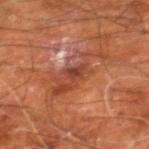Assessment: Part of a total-body skin-imaging series; this lesion was reviewed on a skin check and was not flagged for biopsy. Image and clinical context: A 15 mm close-up extracted from a 3D total-body photography capture. The lesion is located on the right leg. A male patient, aged around 60. The lesion's longest dimension is about 7 mm. Captured under cross-polarized illumination. An algorithmic analysis of the crop reported about 8 CIELAB-L* units darker than the surrounding skin and a lesion-to-skin contrast of about 6.5 (normalized; higher = more distinct). The analysis additionally found a border-irregularity index near 8.5/10, a within-lesion color-variation index near 5/10, and radial color variation of about 1.